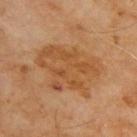Assessment: The lesion was tiled from a total-body skin photograph and was not biopsied. Context: A male subject aged 68–72. A lesion tile, about 15 mm wide, cut from a 3D total-body photograph. The lesion is located on the upper back.